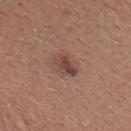biopsy_status: not biopsied; imaged during a skin examination